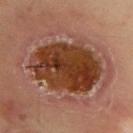Part of a total-body skin-imaging series; this lesion was reviewed on a skin check and was not flagged for biopsy.